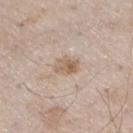{
  "biopsy_status": "not biopsied; imaged during a skin examination",
  "patient": {
    "sex": "male",
    "age_approx": 60
  },
  "lighting": "white-light",
  "image": {
    "source": "total-body photography crop",
    "field_of_view_mm": 15
  },
  "lesion_size": {
    "long_diameter_mm_approx": 3.0
  },
  "site": "right thigh"
}